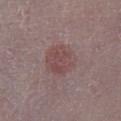No biopsy was performed on this lesion — it was imaged during a full skin examination and was not determined to be concerning.
A 15 mm close-up tile from a total-body photography series done for melanoma screening.
Located on the left lower leg.
The subject is a female about 65 years old.